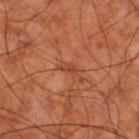Q: Was this lesion biopsied?
A: total-body-photography surveillance lesion; no biopsy
Q: Automated lesion metrics?
A: an average lesion color of about L≈43 a*≈27 b*≈33 (CIELAB), a lesion–skin lightness drop of about 7, and a lesion-to-skin contrast of about 6 (normalized; higher = more distinct); border irregularity of about 3.5 on a 0–10 scale, a within-lesion color-variation index near 0/10, and peripheral color asymmetry of about 0; lesion-presence confidence of about 95/100
Q: Lesion size?
A: ≈2.5 mm
Q: What is the imaging modality?
A: ~15 mm tile from a whole-body skin photo
Q: How was the tile lit?
A: cross-polarized
Q: Patient demographics?
A: male, approximately 70 years of age
Q: Lesion location?
A: the right thigh